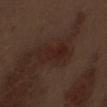Context: This is a white-light tile. The lesion is located on the abdomen. A male patient in their 70s. A roughly 15 mm field-of-view crop from a total-body skin photograph.